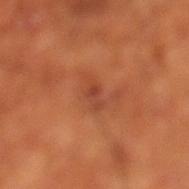Recorded during total-body skin imaging; not selected for excision or biopsy.
A male patient roughly 65 years of age.
From the left lower leg.
Approximately 3 mm at its widest.
This image is a 15 mm lesion crop taken from a total-body photograph.
An algorithmic analysis of the crop reported an area of roughly 3.5 mm². The software also gave a lesion color around L≈43 a*≈28 b*≈34 in CIELAB, about 7 CIELAB-L* units darker than the surrounding skin, and a normalized lesion–skin contrast near 6. The analysis additionally found border irregularity of about 3.5 on a 0–10 scale, a color-variation rating of about 1/10, and a peripheral color-asymmetry measure near 0. The analysis additionally found a nevus-likeness score of about 0/100 and a detector confidence of about 100 out of 100 that the crop contains a lesion.